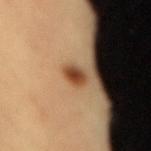subject: female, aged approximately 40 | anatomic site: the lower back | image: total-body-photography crop, ~15 mm field of view | illumination: cross-polarized illumination.